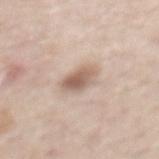Case summary:
• biopsy status: catalogued during a skin exam; not biopsied
• imaging modality: ~15 mm crop, total-body skin-cancer survey
• patient: male, in their mid-50s
• anatomic site: the mid back